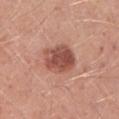The lesion was tiled from a total-body skin photograph and was not biopsied. A male subject aged around 45. On the leg. A close-up tile cropped from a whole-body skin photograph, about 15 mm across.